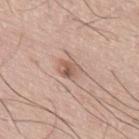Imaged during a routine full-body skin examination; the lesion was not biopsied and no histopathology is available. The subject is a male aged around 75. Automated image analysis of the tile measured a lesion color around L≈58 a*≈19 b*≈27 in CIELAB and roughly 10 lightness units darker than nearby skin. And it measured a border-irregularity rating of about 2/10, a within-lesion color-variation index near 5.5/10, and a peripheral color-asymmetry measure near 2. A region of skin cropped from a whole-body photographic capture, roughly 15 mm wide. Located on the upper back.